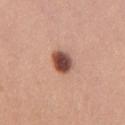{"biopsy_status": "not biopsied; imaged during a skin examination", "lesion_size": {"long_diameter_mm_approx": 3.0}, "patient": {"sex": "female", "age_approx": 55}, "site": "chest", "lighting": "white-light", "image": {"source": "total-body photography crop", "field_of_view_mm": 15}}